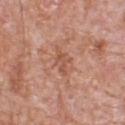Captured during whole-body skin photography for melanoma surveillance; the lesion was not biopsied.
A region of skin cropped from a whole-body photographic capture, roughly 15 mm wide.
On the back.
A male subject approximately 80 years of age.
This is a white-light tile.
The lesion-visualizer software estimated a lesion area of about 4 mm² and a shape-asymmetry score of about 0.3 (0 = symmetric). It also reported internal color variation of about 1.5 on a 0–10 scale and peripheral color asymmetry of about 0.5. The analysis additionally found a nevus-likeness score of about 0/100 and a detector confidence of about 100 out of 100 that the crop contains a lesion.